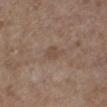notes: total-body-photography surveillance lesion; no biopsy | illumination: white-light | lesion diameter: ~3 mm (longest diameter) | imaging modality: 15 mm crop, total-body photography | subject: female, in their mid- to late 60s | body site: the left lower leg | automated metrics: a mean CIELAB color near L≈47 a*≈15 b*≈26, about 6 CIELAB-L* units darker than the surrounding skin, and a normalized lesion–skin contrast near 5.5; border irregularity of about 3 on a 0–10 scale, a within-lesion color-variation index near 1/10, and peripheral color asymmetry of about 0.5; lesion-presence confidence of about 100/100.